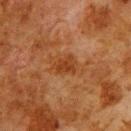Q: How was the tile lit?
A: cross-polarized
Q: What is the imaging modality?
A: ~15 mm crop, total-body skin-cancer survey
Q: Patient demographics?
A: male, approximately 80 years of age
Q: What is the lesion's diameter?
A: ~3 mm (longest diameter)
Q: Automated lesion metrics?
A: a lesion area of about 5 mm² and a shape eccentricity near 0.7; an average lesion color of about L≈32 a*≈22 b*≈32 (CIELAB), roughly 7 lightness units darker than nearby skin, and a lesion-to-skin contrast of about 7 (normalized; higher = more distinct); an automated nevus-likeness rating near 5 out of 100 and lesion-presence confidence of about 100/100
Q: Where on the body is the lesion?
A: the upper back